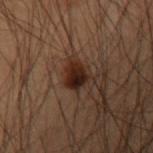biopsy_status: not biopsied; imaged during a skin examination
site: arm
lighting: cross-polarized
patient:
  sex: male
  age_approx: 55
image:
  source: total-body photography crop
  field_of_view_mm: 15
automated_metrics:
  border_irregularity_0_10: 2.5
  color_variation_0_10: 6.0
  peripheral_color_asymmetry: 2.0